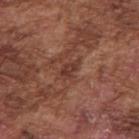Recorded during total-body skin imaging; not selected for excision or biopsy. Captured under white-light illumination. The lesion is on the right upper arm. A roughly 15 mm field-of-view crop from a total-body skin photograph. The subject is a male about 75 years old.